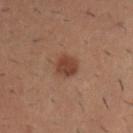Imaged during a routine full-body skin examination; the lesion was not biopsied and no histopathology is available.
This image is a 15 mm lesion crop taken from a total-body photograph.
Approximately 3 mm at its widest.
A male patient, approximately 30 years of age.
From the arm.
Automated tile analysis of the lesion measured a footprint of about 6 mm² and a shape-asymmetry score of about 0.2 (0 = symmetric). The software also gave a lesion–skin lightness drop of about 8 and a lesion-to-skin contrast of about 7.5 (normalized; higher = more distinct). And it measured a border-irregularity index near 1.5/10, a color-variation rating of about 3/10, and radial color variation of about 1.